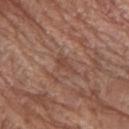biopsy status: no biopsy performed (imaged during a skin exam)
TBP lesion metrics: an area of roughly 2.5 mm², an outline eccentricity of about 0.9 (0 = round, 1 = elongated), and two-axis asymmetry of about 0.45; a lesion color around L≈44 a*≈21 b*≈26 in CIELAB, a lesion–skin lightness drop of about 7, and a normalized border contrast of about 5.5
tile lighting: white-light
location: the left forearm
diameter: about 3 mm
patient: female, about 75 years old
imaging modality: 15 mm crop, total-body photography